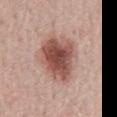The lesion was photographed on a routine skin check and not biopsied; there is no pathology result.
About 5.5 mm across.
A roughly 15 mm field-of-view crop from a total-body skin photograph.
Imaged with white-light lighting.
The subject is a male in their 70s.
The total-body-photography lesion software estimated a lesion–skin lightness drop of about 16 and a normalized lesion–skin contrast near 10.5. The analysis additionally found a color-variation rating of about 5.5/10 and a peripheral color-asymmetry measure near 1.5. And it measured an automated nevus-likeness rating near 95 out of 100 and lesion-presence confidence of about 100/100.
The lesion is on the lower back.